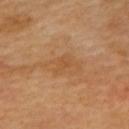{"biopsy_status": "not biopsied; imaged during a skin examination", "patient": {"sex": "female", "age_approx": 60}, "lighting": "cross-polarized", "site": "upper back", "image": {"source": "total-body photography crop", "field_of_view_mm": 15}, "lesion_size": {"long_diameter_mm_approx": 3.0}}